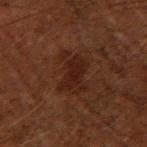biopsy_status: not biopsied; imaged during a skin examination
lighting: cross-polarized
patient:
  sex: male
  age_approx: 50
lesion_size:
  long_diameter_mm_approx: 4.5
site: right upper arm
image:
  source: total-body photography crop
  field_of_view_mm: 15